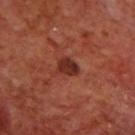No biopsy was performed on this lesion — it was imaged during a full skin examination and was not determined to be concerning.
A male subject, aged around 70.
A 15 mm close-up extracted from a 3D total-body photography capture.
Captured under cross-polarized illumination.
Automated image analysis of the tile measured a border-irregularity index near 2.5/10, a within-lesion color-variation index near 2.5/10, and a peripheral color-asymmetry measure near 1.
The lesion is on the upper back.